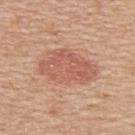Case summary:
- notes: catalogued during a skin exam; not biopsied
- automated lesion analysis: an area of roughly 21 mm² and a shape eccentricity near 0.8; a mean CIELAB color near L≈58 a*≈25 b*≈30, a lesion–skin lightness drop of about 9, and a lesion-to-skin contrast of about 6 (normalized; higher = more distinct); border irregularity of about 2 on a 0–10 scale and internal color variation of about 3 on a 0–10 scale
- image source: ~15 mm tile from a whole-body skin photo
- lesion diameter: about 7 mm
- body site: the back
- patient: female, aged 48–52
- tile lighting: white-light